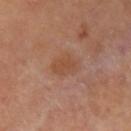notes=imaged on a skin check; not biopsied | size=~3.5 mm (longest diameter) | patient=aged approximately 60 | site=the arm | acquisition=15 mm crop, total-body photography.